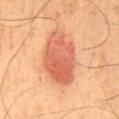Q: Was a biopsy performed?
A: imaged on a skin check; not biopsied
Q: What lighting was used for the tile?
A: cross-polarized
Q: Patient demographics?
A: male, in their mid- to late 40s
Q: What kind of image is this?
A: total-body-photography crop, ~15 mm field of view
Q: What is the lesion's diameter?
A: about 7 mm
Q: What is the anatomic site?
A: the mid back